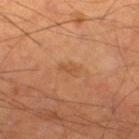workup: catalogued during a skin exam; not biopsied | location: the right thigh | tile lighting: cross-polarized | image source: ~15 mm crop, total-body skin-cancer survey | patient: male, in their mid-60s.